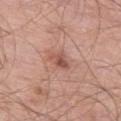A male patient, in their 70s. The total-body-photography lesion software estimated a lesion area of about 4 mm² and a shape eccentricity near 0.75. The analysis additionally found border irregularity of about 3 on a 0–10 scale, a within-lesion color-variation index near 4.5/10, and radial color variation of about 1.5. The analysis additionally found a nevus-likeness score of about 25/100 and a lesion-detection confidence of about 100/100. From the left thigh. Imaged with white-light lighting. A region of skin cropped from a whole-body photographic capture, roughly 15 mm wide. The lesion's longest dimension is about 3 mm.